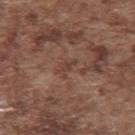biopsy status — total-body-photography surveillance lesion; no biopsy | diameter — ~2.5 mm (longest diameter) | acquisition — 15 mm crop, total-body photography | body site — the upper back | subject — male, aged 73 to 77.